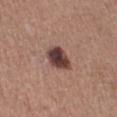Impression:
The lesion was tiled from a total-body skin photograph and was not biopsied.
Image and clinical context:
Imaged with white-light lighting. Cropped from a whole-body photographic skin survey; the tile spans about 15 mm. The recorded lesion diameter is about 3.5 mm. From the right lower leg. A female patient, in their mid- to late 60s. An algorithmic analysis of the crop reported a lesion area of about 8 mm², a shape eccentricity near 0.65, and a shape-asymmetry score of about 0.2 (0 = symmetric). And it measured roughly 17 lightness units darker than nearby skin and a normalized border contrast of about 13. And it measured border irregularity of about 1.5 on a 0–10 scale, internal color variation of about 4.5 on a 0–10 scale, and peripheral color asymmetry of about 1.